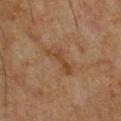Assessment: No biopsy was performed on this lesion — it was imaged during a full skin examination and was not determined to be concerning. Clinical summary: A region of skin cropped from a whole-body photographic capture, roughly 15 mm wide. A male patient approximately 50 years of age. Imaged with cross-polarized lighting. Located on the chest.